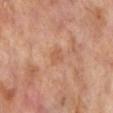workup: no biopsy performed (imaged during a skin exam) | illumination: cross-polarized | location: the left lower leg | subject: male, aged 68 to 72 | automated metrics: a lesion-to-skin contrast of about 5 (normalized; higher = more distinct) | image source: ~15 mm crop, total-body skin-cancer survey.